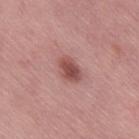The lesion was tiled from a total-body skin photograph and was not biopsied. A female subject roughly 50 years of age. This is a white-light tile. Automated tile analysis of the lesion measured a lesion area of about 6 mm², a shape eccentricity near 0.75, and a shape-asymmetry score of about 0.2 (0 = symmetric). The analysis additionally found about 12 CIELAB-L* units darker than the surrounding skin and a lesion-to-skin contrast of about 8.5 (normalized; higher = more distinct). The lesion is located on the right thigh. The recorded lesion diameter is about 3.5 mm. A 15 mm close-up tile from a total-body photography series done for melanoma screening.